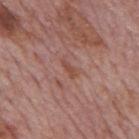This lesion was catalogued during total-body skin photography and was not selected for biopsy. Cropped from a whole-body photographic skin survey; the tile spans about 15 mm. Captured under white-light illumination. A male patient roughly 75 years of age. Longest diameter approximately 2.5 mm. From the mid back. The lesion-visualizer software estimated a border-irregularity rating of about 4.5/10. It also reported an automated nevus-likeness rating near 0 out of 100 and lesion-presence confidence of about 95/100.